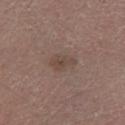Assessment: The lesion was tiled from a total-body skin photograph and was not biopsied. Background: From the leg. Captured under white-light illumination. Cropped from a total-body skin-imaging series; the visible field is about 15 mm. About 3 mm across. The patient is a male aged around 75.